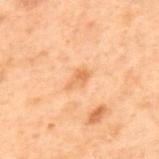workup = total-body-photography surveillance lesion; no biopsy
site = the upper back
subject = male, approximately 70 years of age
image = total-body-photography crop, ~15 mm field of view
automated lesion analysis = a border-irregularity rating of about 2.5/10, a within-lesion color-variation index near 1.5/10, and radial color variation of about 0.5; a nevus-likeness score of about 0/100 and a detector confidence of about 100 out of 100 that the crop contains a lesion